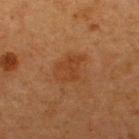  biopsy_status: not biopsied; imaged during a skin examination
  image:
    source: total-body photography crop
    field_of_view_mm: 15
  lesion_size:
    long_diameter_mm_approx: 4.0
  patient:
    sex: male
    age_approx: 65
  site: upper back
  lighting: cross-polarized